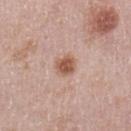Captured during whole-body skin photography for melanoma surveillance; the lesion was not biopsied. This is a white-light tile. Automated image analysis of the tile measured an area of roughly 6 mm², a shape eccentricity near 0.3, and two-axis asymmetry of about 0.25. The software also gave a lesion color around L≈57 a*≈20 b*≈29 in CIELAB. And it measured a border-irregularity rating of about 2/10. The recorded lesion diameter is about 3 mm. A female subject roughly 50 years of age. A close-up tile cropped from a whole-body skin photograph, about 15 mm across. The lesion is located on the left upper arm.